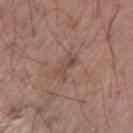| key | value |
|---|---|
| workup | imaged on a skin check; not biopsied |
| subject | male, aged 58–62 |
| site | the arm |
| imaging modality | ~15 mm crop, total-body skin-cancer survey |